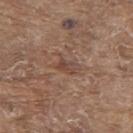• biopsy status · catalogued during a skin exam; not biopsied
• lighting · white-light illumination
• image source · 15 mm crop, total-body photography
• TBP lesion metrics · a border-irregularity rating of about 2.5/10 and a within-lesion color-variation index near 3/10
• patient · male, aged approximately 80
• lesion diameter · ~2.5 mm (longest diameter)
• location · the upper back